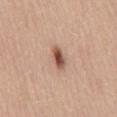Assessment: Recorded during total-body skin imaging; not selected for excision or biopsy. Background: The recorded lesion diameter is about 3 mm. From the mid back. A roughly 15 mm field-of-view crop from a total-body skin photograph. This is a white-light tile. A female subject approximately 60 years of age.